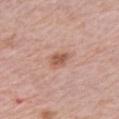<tbp_lesion>
<biopsy_status>not biopsied; imaged during a skin examination</biopsy_status>
</tbp_lesion>